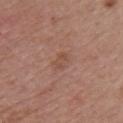This lesion was catalogued during total-body skin photography and was not selected for biopsy.
Measured at roughly 2.5 mm in maximum diameter.
A close-up tile cropped from a whole-body skin photograph, about 15 mm across.
An algorithmic analysis of the crop reported an area of roughly 4 mm², a shape eccentricity near 0.75, and a shape-asymmetry score of about 0.25 (0 = symmetric). The software also gave a border-irregularity rating of about 2.5/10, internal color variation of about 2 on a 0–10 scale, and a peripheral color-asymmetry measure near 0.5. The analysis additionally found an automated nevus-likeness rating near 0 out of 100 and a detector confidence of about 100 out of 100 that the crop contains a lesion.
The subject is a female aged 48 to 52.
The lesion is located on the chest.